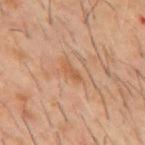| feature | finding |
|---|---|
| biopsy status | imaged on a skin check; not biopsied |
| patient | male, approximately 60 years of age |
| image source | ~15 mm tile from a whole-body skin photo |
| body site | the mid back |
| illumination | cross-polarized |
| lesion diameter | ≈3 mm |